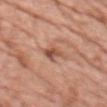biopsy status: imaged on a skin check; not biopsied | automated metrics: a border-irregularity index near 2.5/10, a color-variation rating of about 5.5/10, and radial color variation of about 2 | patient: male, in their mid-70s | acquisition: ~15 mm tile from a whole-body skin photo | body site: the head or neck | size: about 3 mm | lighting: white-light.